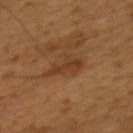Impression:
This lesion was catalogued during total-body skin photography and was not selected for biopsy.
Background:
Imaged with cross-polarized lighting. Measured at roughly 4 mm in maximum diameter. Cropped from a whole-body photographic skin survey; the tile spans about 15 mm. A male subject aged around 45. An algorithmic analysis of the crop reported a footprint of about 6 mm² and an eccentricity of roughly 0.85. The software also gave a mean CIELAB color near L≈35 a*≈20 b*≈31, a lesion–skin lightness drop of about 7, and a lesion-to-skin contrast of about 6 (normalized; higher = more distinct). It also reported border irregularity of about 6 on a 0–10 scale, a color-variation rating of about 2/10, and radial color variation of about 0.5. From the back.